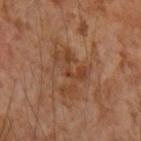workup = total-body-photography surveillance lesion; no biopsy
subject = male, in their mid- to late 50s
location = the right forearm
diameter = about 6 mm
image source = total-body-photography crop, ~15 mm field of view